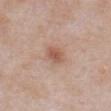notes: imaged on a skin check; not biopsied
location: the abdomen
acquisition: total-body-photography crop, ~15 mm field of view
tile lighting: white-light illumination
diameter: ~2.5 mm (longest diameter)
patient: male, aged around 55
TBP lesion metrics: an average lesion color of about L≈56 a*≈21 b*≈28 (CIELAB), roughly 9 lightness units darker than nearby skin, and a normalized border contrast of about 7; a border-irregularity rating of about 1.5/10, a color-variation rating of about 3/10, and peripheral color asymmetry of about 1